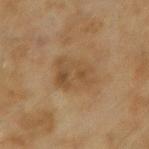Recorded during total-body skin imaging; not selected for excision or biopsy. The lesion is located on the left forearm. A female patient, aged 58–62. Cropped from a whole-body photographic skin survey; the tile spans about 15 mm.